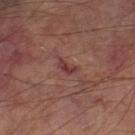workup: imaged on a skin check; not biopsied
image-analysis metrics: a footprint of about 3 mm², an outline eccentricity of about 0.85 (0 = round, 1 = elongated), and a shape-asymmetry score of about 0.35 (0 = symmetric); a lesion color around L≈36 a*≈24 b*≈20 in CIELAB, a lesion–skin lightness drop of about 8, and a normalized lesion–skin contrast near 7.5; a border-irregularity index near 4/10, internal color variation of about 1.5 on a 0–10 scale, and radial color variation of about 0.5; a classifier nevus-likeness of about 0/100
imaging modality: total-body-photography crop, ~15 mm field of view
anatomic site: the left thigh
tile lighting: cross-polarized
lesion size: ~2.5 mm (longest diameter)
subject: male, aged around 70